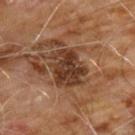This lesion was catalogued during total-body skin photography and was not selected for biopsy. The tile uses cross-polarized illumination. The lesion's longest dimension is about 4.5 mm. The lesion is located on the chest. The patient is a male about 60 years old. A roughly 15 mm field-of-view crop from a total-body skin photograph.